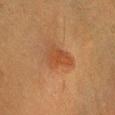Impression: The lesion was photographed on a routine skin check and not biopsied; there is no pathology result. Clinical summary: A close-up tile cropped from a whole-body skin photograph, about 15 mm across. A female subject aged around 55. Located on the head or neck. Imaged with cross-polarized lighting. The lesion's longest dimension is about 4 mm.